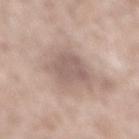This lesion was catalogued during total-body skin photography and was not selected for biopsy. Captured under white-light illumination. Longest diameter approximately 4.5 mm. A male patient approximately 60 years of age. A lesion tile, about 15 mm wide, cut from a 3D total-body photograph. The lesion-visualizer software estimated an area of roughly 12 mm² and an outline eccentricity of about 0.7 (0 = round, 1 = elongated). And it measured a border-irregularity index near 3/10, a within-lesion color-variation index near 2/10, and peripheral color asymmetry of about 0.5. The analysis additionally found a classifier nevus-likeness of about 0/100 and a lesion-detection confidence of about 65/100. On the mid back.